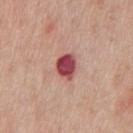This image is a 15 mm lesion crop taken from a total-body photograph. Located on the front of the torso. A male patient, aged approximately 60. Imaged with white-light lighting. The lesion's longest dimension is about 3 mm. The total-body-photography lesion software estimated a border-irregularity rating of about 2/10, internal color variation of about 3.5 on a 0–10 scale, and radial color variation of about 1. And it measured lesion-presence confidence of about 100/100.